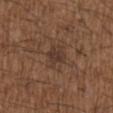Background: A 15 mm close-up extracted from a 3D total-body photography capture. Automated image analysis of the tile measured a mean CIELAB color near L≈35 a*≈17 b*≈24 and a normalized lesion–skin contrast near 7. The patient is a male aged approximately 50. On the chest.